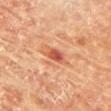Q: Is there a histopathology result?
A: no biopsy performed (imaged during a skin exam)
Q: What are the patient's age and sex?
A: female, roughly 80 years of age
Q: What did automated image analysis measure?
A: a mean CIELAB color near L≈51 a*≈31 b*≈32 and roughly 12 lightness units darker than nearby skin; a border-irregularity index near 2.5/10, internal color variation of about 4.5 on a 0–10 scale, and a peripheral color-asymmetry measure near 1.5
Q: What lighting was used for the tile?
A: cross-polarized illumination
Q: How was this image acquired?
A: ~15 mm tile from a whole-body skin photo
Q: Where on the body is the lesion?
A: the chest
Q: What is the lesion's diameter?
A: about 2.5 mm